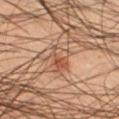Impression:
The lesion was tiled from a total-body skin photograph and was not biopsied.
Context:
From the left thigh. The lesion's longest dimension is about 3.5 mm. This is a cross-polarized tile. A roughly 15 mm field-of-view crop from a total-body skin photograph. The patient is a male aged 43 to 47. Automated image analysis of the tile measured an eccentricity of roughly 0.8 and a symmetry-axis asymmetry near 0.3. The analysis additionally found an average lesion color of about L≈52 a*≈22 b*≈32 (CIELAB), roughly 9 lightness units darker than nearby skin, and a normalized border contrast of about 6.